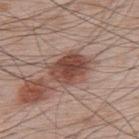notes: total-body-photography surveillance lesion; no biopsy